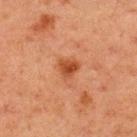notes: total-body-photography surveillance lesion; no biopsy | lesion diameter: about 2.5 mm | acquisition: ~15 mm crop, total-body skin-cancer survey | tile lighting: cross-polarized illumination | patient: male, in their 60s | anatomic site: the upper back | automated lesion analysis: a lesion area of about 4.5 mm², an outline eccentricity of about 0.3 (0 = round, 1 = elongated), and a symmetry-axis asymmetry near 0.35; an automated nevus-likeness rating near 80 out of 100.